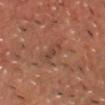Imaged during a routine full-body skin examination; the lesion was not biopsied and no histopathology is available. A male patient aged around 45. Imaged with cross-polarized lighting. The total-body-photography lesion software estimated an outline eccentricity of about 0.9 (0 = round, 1 = elongated) and a symmetry-axis asymmetry near 0.3. A roughly 15 mm field-of-view crop from a total-body skin photograph. On the head or neck. About 3.5 mm across.